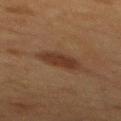Captured during whole-body skin photography for melanoma surveillance; the lesion was not biopsied. Cropped from a whole-body photographic skin survey; the tile spans about 15 mm. Longest diameter approximately 4 mm. From the mid back. The subject is a male roughly 65 years of age. Automated image analysis of the tile measured peripheral color asymmetry of about 0.5. And it measured an automated nevus-likeness rating near 80 out of 100 and a detector confidence of about 100 out of 100 that the crop contains a lesion.